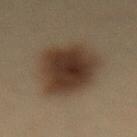Impression: The lesion was tiled from a total-body skin photograph and was not biopsied. Image and clinical context: A female patient aged 28 to 32. Located on the lower back. A lesion tile, about 15 mm wide, cut from a 3D total-body photograph.